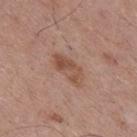| feature | finding |
|---|---|
| notes | total-body-photography surveillance lesion; no biopsy |
| lesion diameter | ≈4.5 mm |
| image | ~15 mm crop, total-body skin-cancer survey |
| subject | male, roughly 70 years of age |
| tile lighting | white-light |
| anatomic site | the back |